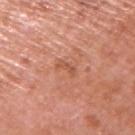A male patient, about 70 years old.
The tile uses white-light illumination.
Cropped from a total-body skin-imaging series; the visible field is about 15 mm.
From the right upper arm.